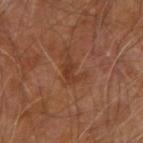Recorded during total-body skin imaging; not selected for excision or biopsy. Cropped from a total-body skin-imaging series; the visible field is about 15 mm. Automated image analysis of the tile measured an area of roughly 4 mm², an outline eccentricity of about 0.8 (0 = round, 1 = elongated), and a shape-asymmetry score of about 0.45 (0 = symmetric). The software also gave a border-irregularity rating of about 6/10, a within-lesion color-variation index near 1/10, and radial color variation of about 0.5. The tile uses cross-polarized illumination. Measured at roughly 3 mm in maximum diameter. The lesion is on the right upper arm. A male subject, in their 70s.